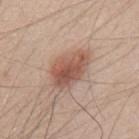Recorded during total-body skin imaging; not selected for excision or biopsy. A close-up tile cropped from a whole-body skin photograph, about 15 mm across. On the back. An algorithmic analysis of the crop reported a lesion-detection confidence of about 100/100. A male patient, in their 50s.